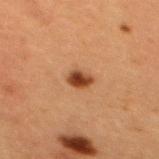No biopsy was performed on this lesion — it was imaged during a full skin examination and was not determined to be concerning.
The patient is a female roughly 40 years of age.
About 2.5 mm across.
The lesion is on the mid back.
A region of skin cropped from a whole-body photographic capture, roughly 15 mm wide.
Imaged with cross-polarized lighting.